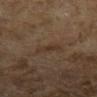The lesion-visualizer software estimated a footprint of about 3 mm², a shape eccentricity near 0.9, and a shape-asymmetry score of about 0.3 (0 = symmetric). The analysis additionally found an average lesion color of about L≈28 a*≈13 b*≈22 (CIELAB) and a lesion-to-skin contrast of about 5.5 (normalized; higher = more distinct). It also reported internal color variation of about 0.5 on a 0–10 scale and a peripheral color-asymmetry measure near 0. The lesion's longest dimension is about 2.5 mm. Cropped from a total-body skin-imaging series; the visible field is about 15 mm. A female patient, aged approximately 60. The tile uses cross-polarized illumination. The lesion is located on the left lower leg.